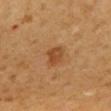follow-up = total-body-photography surveillance lesion; no biopsy | lesion diameter = ≈2.5 mm | subject = male, approximately 60 years of age | body site = the back | image source = total-body-photography crop, ~15 mm field of view | tile lighting = cross-polarized illumination.